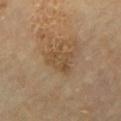The lesion was photographed on a routine skin check and not biopsied; there is no pathology result.
Imaged with cross-polarized lighting.
Automated tile analysis of the lesion measured a mean CIELAB color near L≈47 a*≈15 b*≈32, roughly 7 lightness units darker than nearby skin, and a lesion-to-skin contrast of about 6 (normalized; higher = more distinct). It also reported a border-irregularity rating of about 5/10 and a within-lesion color-variation index near 3/10.
A region of skin cropped from a whole-body photographic capture, roughly 15 mm wide.
The recorded lesion diameter is about 4 mm.
The lesion is located on the chest.
The subject is a male about 70 years old.